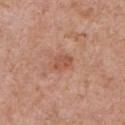This lesion was catalogued during total-body skin photography and was not selected for biopsy. On the chest. A male subject, aged 68–72. A roughly 15 mm field-of-view crop from a total-body skin photograph.